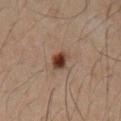follow-up: total-body-photography surveillance lesion; no biopsy
site: the abdomen
automated lesion analysis: a border-irregularity index near 2/10, internal color variation of about 3.5 on a 0–10 scale, and a peripheral color-asymmetry measure near 1; an automated nevus-likeness rating near 100 out of 100
tile lighting: cross-polarized
subject: male, aged 48 to 52
lesion diameter: about 3 mm
image: 15 mm crop, total-body photography